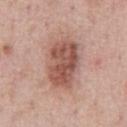Case summary:
• workup: no biopsy performed (imaged during a skin exam)
• site: the chest
• lesion size: ~6.5 mm (longest diameter)
• imaging modality: ~15 mm tile from a whole-body skin photo
• tile lighting: white-light
• subject: male, aged 38–42
• automated lesion analysis: a lesion area of about 19 mm², an outline eccentricity of about 0.8 (0 = round, 1 = elongated), and a shape-asymmetry score of about 0.15 (0 = symmetric); a lesion color around L≈53 a*≈23 b*≈26 in CIELAB, a lesion–skin lightness drop of about 13, and a normalized border contrast of about 9